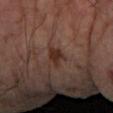biopsy_status: not biopsied; imaged during a skin examination